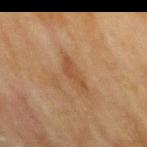{
  "biopsy_status": "not biopsied; imaged during a skin examination",
  "patient": {
    "sex": "male",
    "age_approx": 70
  },
  "image": {
    "source": "total-body photography crop",
    "field_of_view_mm": 15
  },
  "site": "mid back",
  "automated_metrics": {
    "area_mm2_approx": 4.0,
    "eccentricity": 0.95
  },
  "lesion_size": {
    "long_diameter_mm_approx": 4.0
  }
}